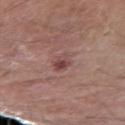Recorded during total-body skin imaging; not selected for excision or biopsy.
The lesion is on the left forearm.
The tile uses white-light illumination.
The patient is a male approximately 80 years of age.
Cropped from a whole-body photographic skin survey; the tile spans about 15 mm.
Measured at roughly 3 mm in maximum diameter.